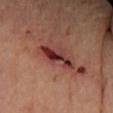About 4.5 mm across. The subject is a female roughly 65 years of age. From the right forearm. The total-body-photography lesion software estimated a border-irregularity index near 4.5/10, a color-variation rating of about 8/10, and a peripheral color-asymmetry measure near 2. It also reported a nevus-likeness score of about 0/100 and lesion-presence confidence of about 80/100. Imaged with cross-polarized lighting. A region of skin cropped from a whole-body photographic capture, roughly 15 mm wide.